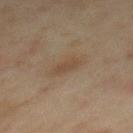Impression:
Captured during whole-body skin photography for melanoma surveillance; the lesion was not biopsied.
Clinical summary:
A 15 mm close-up tile from a total-body photography series done for melanoma screening. On the mid back. A female subject, in their 60s. The tile uses cross-polarized illumination.